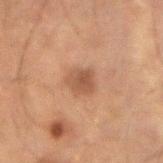An algorithmic analysis of the crop reported an area of roughly 6 mm² and a shape-asymmetry score of about 0.2 (0 = symmetric). And it measured a mean CIELAB color near L≈40 a*≈17 b*≈26, about 8 CIELAB-L* units darker than the surrounding skin, and a normalized border contrast of about 7. It also reported a border-irregularity rating of about 2/10, internal color variation of about 2.5 on a 0–10 scale, and radial color variation of about 1. And it measured a classifier nevus-likeness of about 40/100 and a lesion-detection confidence of about 100/100. The recorded lesion diameter is about 3 mm. A 15 mm close-up tile from a total-body photography series done for melanoma screening. Imaged with cross-polarized lighting. Located on the arm. A male patient about 50 years old.